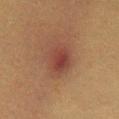Assessment:
Part of a total-body skin-imaging series; this lesion was reviewed on a skin check and was not flagged for biopsy.
Image and clinical context:
This is a cross-polarized tile. The lesion is located on the front of the torso. The subject is a female in their 40s. A 15 mm close-up tile from a total-body photography series done for melanoma screening. Automated image analysis of the tile measured a lesion color around L≈34 a*≈20 b*≈23 in CIELAB and a lesion-to-skin contrast of about 8 (normalized; higher = more distinct). It also reported a border-irregularity rating of about 2/10. And it measured a lesion-detection confidence of about 100/100.